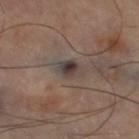Part of a total-body skin-imaging series; this lesion was reviewed on a skin check and was not flagged for biopsy.
A 15 mm close-up extracted from a 3D total-body photography capture.
Located on the right thigh.
The tile uses cross-polarized illumination.